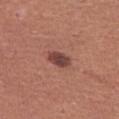{
  "biopsy_status": "not biopsied; imaged during a skin examination",
  "patient": {
    "sex": "female",
    "age_approx": 50
  },
  "image": {
    "source": "total-body photography crop",
    "field_of_view_mm": 15
  },
  "site": "leg",
  "lighting": "white-light",
  "lesion_size": {
    "long_diameter_mm_approx": 3.0
  }
}